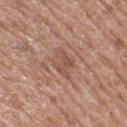Q: Was a biopsy performed?
A: catalogued during a skin exam; not biopsied
Q: What did automated image analysis measure?
A: an automated nevus-likeness rating near 0 out of 100 and lesion-presence confidence of about 90/100
Q: Who is the patient?
A: male, aged around 80
Q: Lesion location?
A: the left lower leg
Q: What kind of image is this?
A: total-body-photography crop, ~15 mm field of view
Q: How large is the lesion?
A: ~3.5 mm (longest diameter)
Q: What lighting was used for the tile?
A: white-light